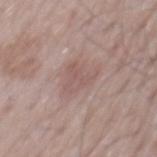<lesion>
  <image>
    <source>total-body photography crop</source>
    <field_of_view_mm>15</field_of_view_mm>
  </image>
  <site>back</site>
  <lesion_size>
    <long_diameter_mm_approx>4.0</long_diameter_mm_approx>
  </lesion_size>
  <patient>
    <sex>male</sex>
    <age_approx>65</age_approx>
  </patient>
  <lighting>white-light</lighting>
</lesion>